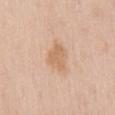| field | value |
|---|---|
| notes | total-body-photography surveillance lesion; no biopsy |
| body site | the mid back |
| illumination | white-light illumination |
| automated lesion analysis | a footprint of about 7.5 mm², an outline eccentricity of about 0.7 (0 = round, 1 = elongated), and a shape-asymmetry score of about 0.35 (0 = symmetric); roughly 8 lightness units darker than nearby skin; a border-irregularity index near 3.5/10, internal color variation of about 2 on a 0–10 scale, and a peripheral color-asymmetry measure near 0.5 |
| image source | 15 mm crop, total-body photography |
| lesion size | ≈4 mm |
| subject | male, approximately 60 years of age |